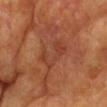lighting: cross-polarized
lesion_size:
  long_diameter_mm_approx: 5.0
image:
  source: total-body photography crop
  field_of_view_mm: 15
patient:
  sex: female
  age_approx: 75
site: head or neck